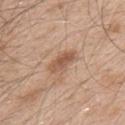{"lighting": "white-light", "automated_metrics": {"area_mm2_approx": 6.0, "eccentricity": 0.85, "shape_asymmetry": 0.2, "nevus_likeness_0_100": 40, "lesion_detection_confidence_0_100": 100}, "patient": {"sex": "male", "age_approx": 50}, "lesion_size": {"long_diameter_mm_approx": 3.5}, "site": "left upper arm", "image": {"source": "total-body photography crop", "field_of_view_mm": 15}}